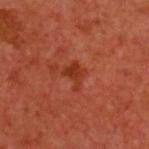No biopsy was performed on this lesion — it was imaged during a full skin examination and was not determined to be concerning. The tile uses cross-polarized illumination. A roughly 15 mm field-of-view crop from a total-body skin photograph. The lesion is on the upper back. The lesion's longest dimension is about 2.5 mm. The patient is a male roughly 60 years of age.